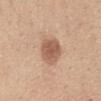A 15 mm close-up tile from a total-body photography series done for melanoma screening. The lesion is on the chest. A female subject aged 63–67.